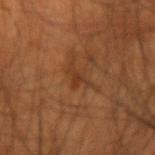{"biopsy_status": "not biopsied; imaged during a skin examination", "site": "right forearm", "patient": {"sex": "male", "age_approx": 55}, "image": {"source": "total-body photography crop", "field_of_view_mm": 15}}